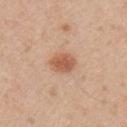Part of a total-body skin-imaging series; this lesion was reviewed on a skin check and was not flagged for biopsy. A male subject, aged around 30. From the chest. The lesion-visualizer software estimated a lesion area of about 6.5 mm². It also reported an average lesion color of about L≈58 a*≈23 b*≈33 (CIELAB). The analysis additionally found a border-irregularity index near 2/10, a within-lesion color-variation index near 2.5/10, and a peripheral color-asymmetry measure near 1. And it measured an automated nevus-likeness rating near 95 out of 100 and a lesion-detection confidence of about 100/100. Cropped from a total-body skin-imaging series; the visible field is about 15 mm. Imaged with white-light lighting.